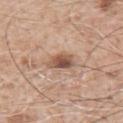The lesion's longest dimension is about 3.5 mm. The lesion is on the arm. Imaged with white-light lighting. The total-body-photography lesion software estimated a lesion area of about 6 mm² and a shape eccentricity near 0.8. The analysis additionally found a normalized lesion–skin contrast near 9. And it measured border irregularity of about 3 on a 0–10 scale, internal color variation of about 6 on a 0–10 scale, and peripheral color asymmetry of about 2. A male patient aged approximately 60. Cropped from a total-body skin-imaging series; the visible field is about 15 mm.